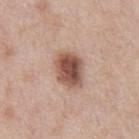{"biopsy_status": "not biopsied; imaged during a skin examination", "patient": {"sex": "male", "age_approx": 60}, "site": "abdomen", "lesion_size": {"long_diameter_mm_approx": 4.5}, "image": {"source": "total-body photography crop", "field_of_view_mm": 15}}